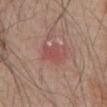Impression: This lesion was catalogued during total-body skin photography and was not selected for biopsy. Background: Cropped from a whole-body photographic skin survey; the tile spans about 15 mm. The subject is a male aged around 80. The lesion is located on the abdomen.